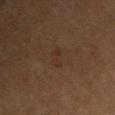Case summary:
– follow-up — total-body-photography surveillance lesion; no biopsy
– lighting — cross-polarized illumination
– acquisition — 15 mm crop, total-body photography
– anatomic site — the front of the torso
– lesion diameter — about 2.5 mm
– patient — female, aged approximately 65